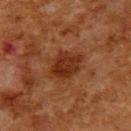notes: total-body-photography surveillance lesion; no biopsy
subject: male, aged approximately 80
diameter: ~4.5 mm (longest diameter)
acquisition: 15 mm crop, total-body photography
anatomic site: the upper back
illumination: cross-polarized